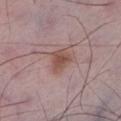{
  "biopsy_status": "not biopsied; imaged during a skin examination",
  "automated_metrics": {
    "area_mm2_approx": 6.0,
    "cielab_L": 50,
    "cielab_a": 20,
    "cielab_b": 23,
    "vs_skin_darker_L": 9.0,
    "vs_skin_contrast_norm": 7.5,
    "nevus_likeness_0_100": 90,
    "lesion_detection_confidence_0_100": 100
  },
  "image": {
    "source": "total-body photography crop",
    "field_of_view_mm": 15
  },
  "lighting": "white-light",
  "patient": {
    "sex": "male",
    "age_approx": 50
  },
  "lesion_size": {
    "long_diameter_mm_approx": 3.0
  },
  "site": "left lower leg"
}